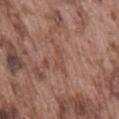- notes: imaged on a skin check; not biopsied